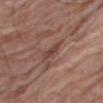Assessment: This lesion was catalogued during total-body skin photography and was not selected for biopsy. Clinical summary: A close-up tile cropped from a whole-body skin photograph, about 15 mm across. From the right thigh. This is a white-light tile. A female subject, roughly 80 years of age. The recorded lesion diameter is about 3.5 mm.